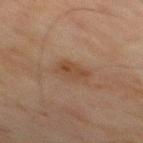Captured during whole-body skin photography for melanoma surveillance; the lesion was not biopsied.
Measured at roughly 3 mm in maximum diameter.
Imaged with cross-polarized lighting.
A 15 mm close-up extracted from a 3D total-body photography capture.
Automated tile analysis of the lesion measured an average lesion color of about L≈37 a*≈16 b*≈26 (CIELAB) and a lesion-to-skin contrast of about 6.5 (normalized; higher = more distinct). The analysis additionally found a border-irregularity index near 2.5/10, internal color variation of about 3.5 on a 0–10 scale, and a peripheral color-asymmetry measure near 1.
From the chest.
The subject is a male roughly 70 years of age.